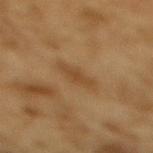| feature | finding |
|---|---|
| notes | imaged on a skin check; not biopsied |
| subject | male, about 85 years old or older |
| anatomic site | the mid back |
| image source | ~15 mm crop, total-body skin-cancer survey |
| lighting | cross-polarized illumination |